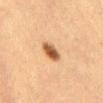{"biopsy_status": "not biopsied; imaged during a skin examination", "automated_metrics": {"shape_asymmetry": 0.25, "border_irregularity_0_10": 2.0, "peripheral_color_asymmetry": 1.5, "nevus_likeness_0_100": 100, "lesion_detection_confidence_0_100": 100}, "image": {"source": "total-body photography crop", "field_of_view_mm": 15}, "patient": {"sex": "female", "age_approx": 40}, "site": "abdomen", "lighting": "cross-polarized"}